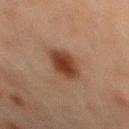The lesion was photographed on a routine skin check and not biopsied; there is no pathology result. A 15 mm close-up extracted from a 3D total-body photography capture. The subject is a male aged approximately 70. The lesion is on the abdomen.